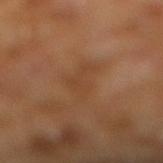Part of a total-body skin-imaging series; this lesion was reviewed on a skin check and was not flagged for biopsy.
This is a cross-polarized tile.
A male subject roughly 60 years of age.
The recorded lesion diameter is about 4.5 mm.
An algorithmic analysis of the crop reported an average lesion color of about L≈39 a*≈19 b*≈31 (CIELAB), about 5 CIELAB-L* units darker than the surrounding skin, and a lesion-to-skin contrast of about 4.5 (normalized; higher = more distinct). The software also gave an automated nevus-likeness rating near 0 out of 100 and a detector confidence of about 100 out of 100 that the crop contains a lesion.
A 15 mm close-up tile from a total-body photography series done for melanoma screening.
Located on the right lower leg.